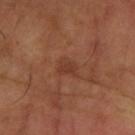No biopsy was performed on this lesion — it was imaged during a full skin examination and was not determined to be concerning. Longest diameter approximately 2.5 mm. Cropped from a total-body skin-imaging series; the visible field is about 15 mm. Automated tile analysis of the lesion measured a footprint of about 4 mm², a shape eccentricity near 0.6, and a shape-asymmetry score of about 0.35 (0 = symmetric). And it measured a mean CIELAB color near L≈38 a*≈23 b*≈30 and a normalized border contrast of about 5.5. It also reported border irregularity of about 3 on a 0–10 scale and a color-variation rating of about 2/10. The patient is a male aged 63–67. The lesion is located on the left forearm.